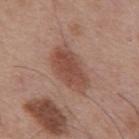Imaged during a routine full-body skin examination; the lesion was not biopsied and no histopathology is available. Captured under white-light illumination. On the mid back. A 15 mm close-up tile from a total-body photography series done for melanoma screening. Approximately 6 mm at its widest. A male patient, aged approximately 55.